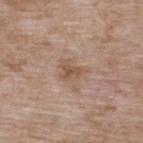| key | value |
|---|---|
| notes | catalogued during a skin exam; not biopsied |
| imaging modality | ~15 mm tile from a whole-body skin photo |
| illumination | white-light illumination |
| anatomic site | the upper back |
| lesion size | ~3 mm (longest diameter) |
| patient | female, approximately 75 years of age |
| TBP lesion metrics | a mean CIELAB color near L≈54 a*≈17 b*≈28, about 8 CIELAB-L* units darker than the surrounding skin, and a normalized border contrast of about 6; a border-irregularity rating of about 4.5/10, a color-variation rating of about 2.5/10, and a peripheral color-asymmetry measure near 1 |